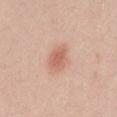Clinical impression:
No biopsy was performed on this lesion — it was imaged during a full skin examination and was not determined to be concerning.
Context:
Imaged with white-light lighting. A region of skin cropped from a whole-body photographic capture, roughly 15 mm wide. The lesion is located on the left thigh. A female subject in their 20s.